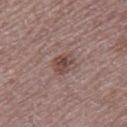Imaged during a routine full-body skin examination; the lesion was not biopsied and no histopathology is available.
Automated tile analysis of the lesion measured border irregularity of about 2.5 on a 0–10 scale, a color-variation rating of about 4.5/10, and peripheral color asymmetry of about 1.5.
Captured under white-light illumination.
A male subject roughly 75 years of age.
A lesion tile, about 15 mm wide, cut from a 3D total-body photograph.
The lesion is on the right thigh.
Approximately 3 mm at its widest.